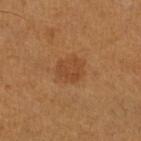follow-up = no biopsy performed (imaged during a skin exam) | automated metrics = a footprint of about 7 mm² and an eccentricity of roughly 0.5 | location = the right lower leg | patient = male, in their mid-50s | acquisition = ~15 mm tile from a whole-body skin photo | lighting = cross-polarized | size = ~3 mm (longest diameter).